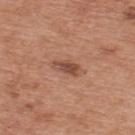No biopsy was performed on this lesion — it was imaged during a full skin examination and was not determined to be concerning. A 15 mm close-up tile from a total-body photography series done for melanoma screening. From the upper back. A male patient, aged 68–72. Automated image analysis of the tile measured a mean CIELAB color near L≈49 a*≈23 b*≈29 and a normalized lesion–skin contrast near 7.5. It also reported a border-irregularity index near 2/10 and peripheral color asymmetry of about 1.5. The tile uses white-light illumination.